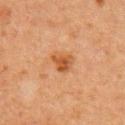Imaged during a routine full-body skin examination; the lesion was not biopsied and no histopathology is available. The lesion is on the right upper arm. This is a cross-polarized tile. A female patient aged approximately 40. Automated tile analysis of the lesion measured a lesion area of about 4.5 mm², an outline eccentricity of about 0.45 (0 = round, 1 = elongated), and two-axis asymmetry of about 0.3. The software also gave an average lesion color of about L≈45 a*≈23 b*≈36 (CIELAB) and a normalized lesion–skin contrast near 8. And it measured a detector confidence of about 100 out of 100 that the crop contains a lesion. A close-up tile cropped from a whole-body skin photograph, about 15 mm across.